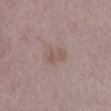From the left lower leg.
A female patient, aged 58 to 62.
Cropped from a whole-body photographic skin survey; the tile spans about 15 mm.